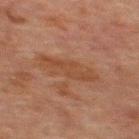size: ~5.5 mm (longest diameter) | anatomic site: the mid back | TBP lesion metrics: a lesion color around L≈34 a*≈18 b*≈26 in CIELAB, a lesion–skin lightness drop of about 5, and a lesion-to-skin contrast of about 6 (normalized; higher = more distinct) | acquisition: 15 mm crop, total-body photography | subject: male, roughly 60 years of age.